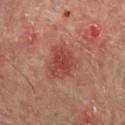Notes:
* notes: total-body-photography surveillance lesion; no biopsy
* body site: the mid back
* image: ~15 mm crop, total-body skin-cancer survey
* illumination: cross-polarized illumination
* patient: male, aged around 65